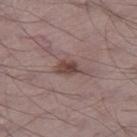Captured during whole-body skin photography for melanoma surveillance; the lesion was not biopsied. A male subject aged 68–72. Automated tile analysis of the lesion measured a border-irregularity rating of about 2.5/10 and internal color variation of about 4 on a 0–10 scale. Captured under white-light illumination. A 15 mm close-up tile from a total-body photography series done for melanoma screening. The lesion is located on the leg. The recorded lesion diameter is about 3 mm.